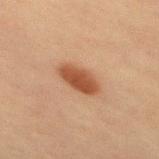Q: Is there a histopathology result?
A: no biopsy performed (imaged during a skin exam)
Q: What is the anatomic site?
A: the back
Q: How was this image acquired?
A: ~15 mm crop, total-body skin-cancer survey
Q: Automated lesion metrics?
A: an outline eccentricity of about 0.8 (0 = round, 1 = elongated) and a shape-asymmetry score of about 0.15 (0 = symmetric); a mean CIELAB color near L≈42 a*≈22 b*≈30, roughly 12 lightness units darker than nearby skin, and a normalized border contrast of about 9.5; border irregularity of about 1.5 on a 0–10 scale, internal color variation of about 2.5 on a 0–10 scale, and a peripheral color-asymmetry measure near 1
Q: What is the lesion's diameter?
A: about 4 mm
Q: What are the patient's age and sex?
A: male, aged around 60